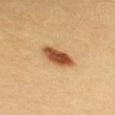Q: Who is the patient?
A: female, aged 28–32
Q: What is the imaging modality?
A: 15 mm crop, total-body photography
Q: Where on the body is the lesion?
A: the chest